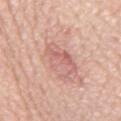This lesion was catalogued during total-body skin photography and was not selected for biopsy. A 15 mm crop from a total-body photograph taken for skin-cancer surveillance. The tile uses white-light illumination. A male patient, about 70 years old. Measured at roughly 5 mm in maximum diameter. The lesion is on the chest.